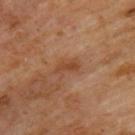Part of a total-body skin-imaging series; this lesion was reviewed on a skin check and was not flagged for biopsy. A roughly 15 mm field-of-view crop from a total-body skin photograph. A male subject aged approximately 60. Located on the back.